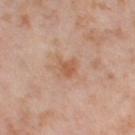Captured during whole-body skin photography for melanoma surveillance; the lesion was not biopsied. A close-up tile cropped from a whole-body skin photograph, about 15 mm across. A female subject approximately 55 years of age. This is a cross-polarized tile. About 2.5 mm across. From the right thigh.